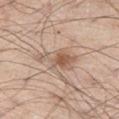follow-up: no biopsy performed (imaged during a skin exam)
patient: male, aged 58–62
body site: the left thigh
image: ~15 mm tile from a whole-body skin photo
illumination: white-light illumination